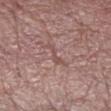The lesion was photographed on a routine skin check and not biopsied; there is no pathology result. An algorithmic analysis of the crop reported a border-irregularity rating of about 6/10, a within-lesion color-variation index near 0/10, and a peripheral color-asymmetry measure near 0. The lesion's longest dimension is about 3 mm. Located on the right forearm. Cropped from a whole-body photographic skin survey; the tile spans about 15 mm. A male patient, aged 68 to 72. The tile uses white-light illumination.